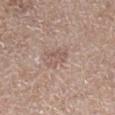Findings:
– follow-up — catalogued during a skin exam; not biopsied
– tile lighting — white-light illumination
– patient — male, about 65 years old
– image — total-body-photography crop, ~15 mm field of view
– lesion diameter — ~3 mm (longest diameter)
– body site — the right lower leg